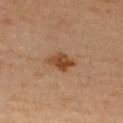follow-up: no biopsy performed (imaged during a skin exam); image: 15 mm crop, total-body photography; size: ~3 mm (longest diameter); location: the upper back; patient: female, about 40 years old; TBP lesion metrics: an outline eccentricity of about 0.7 (0 = round, 1 = elongated) and a symmetry-axis asymmetry near 0.3; illumination: cross-polarized illumination.